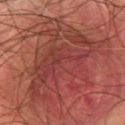Impression:
The lesion was tiled from a total-body skin photograph and was not biopsied.
Acquisition and patient details:
A region of skin cropped from a whole-body photographic capture, roughly 15 mm wide. Imaged with cross-polarized lighting. Longest diameter approximately 11 mm. The patient is a male in their mid- to late 40s. From the front of the torso.